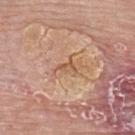Case summary:
– lesion size · about 2.5 mm
– acquisition · total-body-photography crop, ~15 mm field of view
– lighting · white-light
– body site · the chest
– subject · female, aged 63 to 67